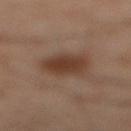{"biopsy_status": "not biopsied; imaged during a skin examination", "patient": {"sex": "male", "age_approx": 55}, "site": "right lower leg", "lighting": "cross-polarized", "automated_metrics": {"border_irregularity_0_10": 2.0, "color_variation_0_10": 3.0, "nevus_likeness_0_100": 100, "lesion_detection_confidence_0_100": 100}, "image": {"source": "total-body photography crop", "field_of_view_mm": 15}}